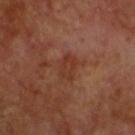Imaged with cross-polarized lighting.
From the head or neck.
Automated tile analysis of the lesion measured a footprint of about 5.5 mm². And it measured about 6 CIELAB-L* units darker than the surrounding skin and a normalized border contrast of about 5.5.
A male subject, about 60 years old.
A 15 mm crop from a total-body photograph taken for skin-cancer surveillance.
The lesion's longest dimension is about 3.5 mm.